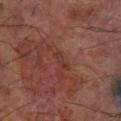Part of a total-body skin-imaging series; this lesion was reviewed on a skin check and was not flagged for biopsy. Cropped from a total-body skin-imaging series; the visible field is about 15 mm. The subject is a male about 65 years old. Captured under cross-polarized illumination. About 2.5 mm across. On the right forearm.